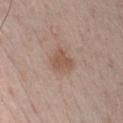Assessment: This lesion was catalogued during total-body skin photography and was not selected for biopsy. Clinical summary: A 15 mm close-up extracted from a 3D total-body photography capture. A male subject, in their mid-50s. The recorded lesion diameter is about 3 mm. The lesion is on the chest. Captured under white-light illumination.